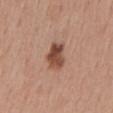Longest diameter approximately 3.5 mm. The lesion is on the back. A region of skin cropped from a whole-body photographic capture, roughly 15 mm wide. The patient is a female aged approximately 55. The lesion-visualizer software estimated a mean CIELAB color near L≈48 a*≈22 b*≈28, about 14 CIELAB-L* units darker than the surrounding skin, and a normalized border contrast of about 10.